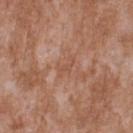Captured during whole-body skin photography for melanoma surveillance; the lesion was not biopsied.
The lesion is on the upper back.
A male subject in their mid-40s.
A 15 mm close-up tile from a total-body photography series done for melanoma screening.